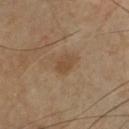Findings:
- site: the chest
- image source: 15 mm crop, total-body photography
- subject: male, aged approximately 45